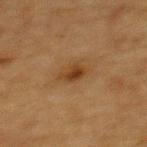Clinical impression:
Imaged during a routine full-body skin examination; the lesion was not biopsied and no histopathology is available.
Acquisition and patient details:
The lesion-visualizer software estimated an area of roughly 4 mm² and a shape-asymmetry score of about 0.35 (0 = symmetric). And it measured a within-lesion color-variation index near 3/10. This image is a 15 mm lesion crop taken from a total-body photograph. Located on the back. A female subject aged 53–57.